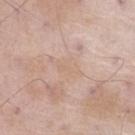tile lighting=white-light illumination
diameter=~2.5 mm (longest diameter)
TBP lesion metrics=an area of roughly 3.5 mm² and a symmetry-axis asymmetry near 0.35; border irregularity of about 4 on a 0–10 scale, internal color variation of about 0.5 on a 0–10 scale, and peripheral color asymmetry of about 0
anatomic site=the leg
image=~15 mm crop, total-body skin-cancer survey
subject=male, approximately 55 years of age